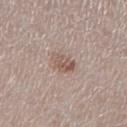biopsy status: total-body-photography surveillance lesion; no biopsy
TBP lesion metrics: a mean CIELAB color near L≈55 a*≈16 b*≈24
lesion diameter: about 3 mm
body site: the right lower leg
illumination: white-light
subject: female, aged 48 to 52
image: ~15 mm tile from a whole-body skin photo